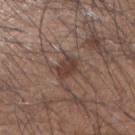Recorded during total-body skin imaging; not selected for excision or biopsy. A male patient, about 35 years old. This image is a 15 mm lesion crop taken from a total-body photograph. The lesion is located on the right forearm.